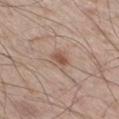Impression:
Imaged during a routine full-body skin examination; the lesion was not biopsied and no histopathology is available.
Acquisition and patient details:
A roughly 15 mm field-of-view crop from a total-body skin photograph. Located on the right thigh. A male subject, approximately 60 years of age.